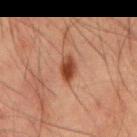No biopsy was performed on this lesion — it was imaged during a full skin examination and was not determined to be concerning. From the mid back. A 15 mm close-up extracted from a 3D total-body photography capture. A male patient, aged 48 to 52.